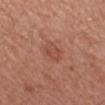notes = total-body-photography surveillance lesion; no biopsy
image = 15 mm crop, total-body photography
tile lighting = white-light illumination
patient = female, aged approximately 50
automated metrics = a footprint of about 5.5 mm², a shape eccentricity near 0.7, and two-axis asymmetry of about 0.2; a mean CIELAB color near L≈49 a*≈25 b*≈29, a lesion–skin lightness drop of about 7, and a normalized lesion–skin contrast near 5
site = the left forearm